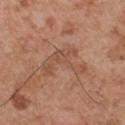follow-up: catalogued during a skin exam; not biopsied | imaging modality: 15 mm crop, total-body photography | anatomic site: the arm | patient: male, aged around 55.